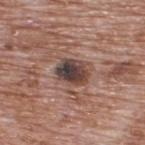Part of a total-body skin-imaging series; this lesion was reviewed on a skin check and was not flagged for biopsy. The patient is a male aged around 70. The lesion-visualizer software estimated a mean CIELAB color near L≈41 a*≈16 b*≈20, roughly 15 lightness units darker than nearby skin, and a lesion-to-skin contrast of about 12 (normalized; higher = more distinct). On the upper back. Longest diameter approximately 3.5 mm. A roughly 15 mm field-of-view crop from a total-body skin photograph.